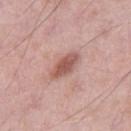This lesion was catalogued during total-body skin photography and was not selected for biopsy. Located on the left thigh. A male subject, in their mid- to late 50s. The tile uses white-light illumination. A lesion tile, about 15 mm wide, cut from a 3D total-body photograph. Automated image analysis of the tile measured a lesion area of about 7 mm² and a symmetry-axis asymmetry near 0.25. It also reported an average lesion color of about L≈55 a*≈23 b*≈25 (CIELAB), about 12 CIELAB-L* units darker than the surrounding skin, and a lesion-to-skin contrast of about 8 (normalized; higher = more distinct). The software also gave border irregularity of about 2.5 on a 0–10 scale, a color-variation rating of about 3/10, and peripheral color asymmetry of about 1. The analysis additionally found a nevus-likeness score of about 60/100 and a detector confidence of about 100 out of 100 that the crop contains a lesion. Approximately 4 mm at its widest.